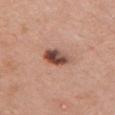{"biopsy_status": "not biopsied; imaged during a skin examination", "automated_metrics": {"vs_skin_darker_L": 16.0, "vs_skin_contrast_norm": 11.0, "nevus_likeness_0_100": 75}, "lesion_size": {"long_diameter_mm_approx": 3.5}, "image": {"source": "total-body photography crop", "field_of_view_mm": 15}, "site": "chest", "patient": {"sex": "male", "age_approx": 60}}